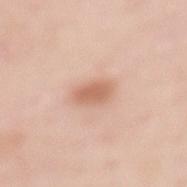Recorded during total-body skin imaging; not selected for excision or biopsy. The patient is a female about 50 years old. The lesion is located on the mid back. This is a white-light tile. This image is a 15 mm lesion crop taken from a total-body photograph. Longest diameter approximately 3.5 mm.